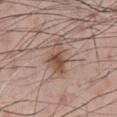The lesion was photographed on a routine skin check and not biopsied; there is no pathology result.
Approximately 5 mm at its widest.
Imaged with white-light lighting.
Cropped from a whole-body photographic skin survey; the tile spans about 15 mm.
A male patient aged 58 to 62.
The lesion is located on the chest.